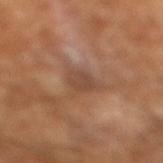follow-up = total-body-photography surveillance lesion; no biopsy | illumination = cross-polarized | acquisition = ~15 mm tile from a whole-body skin photo | size = ~2.5 mm (longest diameter) | patient = male, approximately 65 years of age.